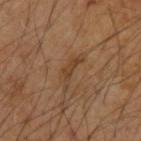<lesion>
<lesion_size>
  <long_diameter_mm_approx>4.5</long_diameter_mm_approx>
</lesion_size>
<site>upper back</site>
<lighting>cross-polarized</lighting>
<patient>
  <sex>male</sex>
  <age_approx>55</age_approx>
</patient>
<image>
  <source>total-body photography crop</source>
  <field_of_view_mm>15</field_of_view_mm>
</image>
</lesion>